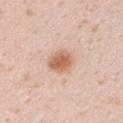The lesion was tiled from a total-body skin photograph and was not biopsied. A lesion tile, about 15 mm wide, cut from a 3D total-body photograph. Located on the right upper arm. The patient is a male in their mid- to late 30s. Imaged with white-light lighting.